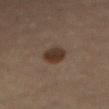Recorded during total-body skin imaging; not selected for excision or biopsy.
A female subject, approximately 65 years of age.
A 15 mm close-up tile from a total-body photography series done for melanoma screening.
Located on the abdomen.
This is a cross-polarized tile.